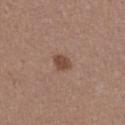workup: no biopsy performed (imaged during a skin exam)
lighting: white-light
diameter: ~2.5 mm (longest diameter)
subject: female, in their mid-30s
acquisition: total-body-photography crop, ~15 mm field of view
location: the right thigh